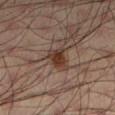Q: Was this lesion biopsied?
A: no biopsy performed (imaged during a skin exam)
Q: What lighting was used for the tile?
A: cross-polarized
Q: What did automated image analysis measure?
A: a lesion area of about 5 mm²; internal color variation of about 3 on a 0–10 scale and peripheral color asymmetry of about 1
Q: What is the imaging modality?
A: total-body-photography crop, ~15 mm field of view
Q: Who is the patient?
A: male, aged 33 to 37
Q: Lesion location?
A: the leg